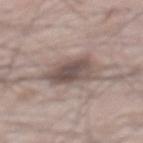The lesion was tiled from a total-body skin photograph and was not biopsied.
Located on the mid back.
Approximately 5 mm at its widest.
A roughly 15 mm field-of-view crop from a total-body skin photograph.
The subject is a male aged 63–67.
Automated tile analysis of the lesion measured an average lesion color of about L≈51 a*≈13 b*≈19 (CIELAB) and a normalized border contrast of about 8.5. And it measured border irregularity of about 3 on a 0–10 scale, a color-variation rating of about 4.5/10, and a peripheral color-asymmetry measure near 1.5.